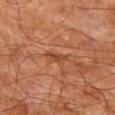Assessment:
Captured during whole-body skin photography for melanoma surveillance; the lesion was not biopsied.
Clinical summary:
The recorded lesion diameter is about 2.5 mm. On the left thigh. A roughly 15 mm field-of-view crop from a total-body skin photograph. A male subject, aged around 80. Automated tile analysis of the lesion measured a lesion area of about 2.5 mm², an eccentricity of roughly 0.95, and a symmetry-axis asymmetry near 0.4. And it measured a lesion color around L≈34 a*≈20 b*≈27 in CIELAB and a lesion-to-skin contrast of about 6.5 (normalized; higher = more distinct). It also reported a border-irregularity rating of about 4/10, a color-variation rating of about 0/10, and peripheral color asymmetry of about 0.